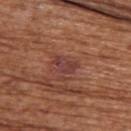This is a white-light tile. Automated image analysis of the tile measured a mean CIELAB color near L≈40 a*≈25 b*≈23 and a lesion-to-skin contrast of about 7.5 (normalized; higher = more distinct). And it measured border irregularity of about 3.5 on a 0–10 scale and internal color variation of about 2.5 on a 0–10 scale. And it measured a nevus-likeness score of about 5/100 and a lesion-detection confidence of about 100/100. The subject is a female about 80 years old. The lesion's longest dimension is about 3 mm. A 15 mm close-up tile from a total-body photography series done for melanoma screening. The lesion is on the upper back.